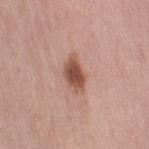Imaged during a routine full-body skin examination; the lesion was not biopsied and no histopathology is available.
Cropped from a whole-body photographic skin survey; the tile spans about 15 mm.
A female patient, approximately 50 years of age.
From the leg.